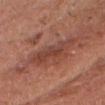The lesion was tiled from a total-body skin photograph and was not biopsied. An algorithmic analysis of the crop reported an outline eccentricity of about 0.95 (0 = round, 1 = elongated). The software also gave a border-irregularity rating of about 5/10 and a peripheral color-asymmetry measure near 1.5. And it measured a nevus-likeness score of about 0/100 and lesion-presence confidence of about 55/100. A close-up tile cropped from a whole-body skin photograph, about 15 mm across. This is a white-light tile. The patient is a male aged around 60. The lesion is on the head or neck.